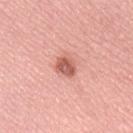Q: Was this lesion biopsied?
A: imaged on a skin check; not biopsied
Q: Lesion size?
A: about 2.5 mm
Q: Illumination type?
A: white-light
Q: What is the anatomic site?
A: the lower back
Q: What is the imaging modality?
A: ~15 mm tile from a whole-body skin photo
Q: Patient demographics?
A: female, in their 70s